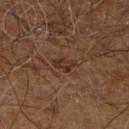Part of a total-body skin-imaging series; this lesion was reviewed on a skin check and was not flagged for biopsy.
The lesion is located on the leg.
The lesion's longest dimension is about 3 mm.
A male subject, aged 58 to 62.
Imaged with cross-polarized lighting.
A 15 mm crop from a total-body photograph taken for skin-cancer surveillance.